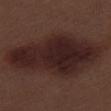Acquisition and patient details: The subject is a male about 70 years old. The lesion is on the leg. Cropped from a whole-body photographic skin survey; the tile spans about 15 mm. An algorithmic analysis of the crop reported an area of roughly 55 mm², an outline eccentricity of about 0.9 (0 = round, 1 = elongated), and a symmetry-axis asymmetry near 0.25. The analysis additionally found a lesion color around L≈23 a*≈18 b*≈17 in CIELAB, about 10 CIELAB-L* units darker than the surrounding skin, and a normalized border contrast of about 11.5. And it measured border irregularity of about 3.5 on a 0–10 scale, a color-variation rating of about 4.5/10, and a peripheral color-asymmetry measure near 1.5. About 14 mm across.